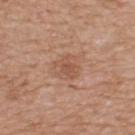Clinical summary: The subject is a male aged 53–57. A lesion tile, about 15 mm wide, cut from a 3D total-body photograph. This is a white-light tile. The lesion is located on the upper back.